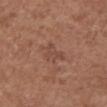workup — total-body-photography surveillance lesion; no biopsy | site — the chest | subject — female, aged around 75 | lesion diameter — about 3 mm | image — ~15 mm crop, total-body skin-cancer survey | lighting — white-light.